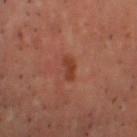Findings:
* notes: no biopsy performed (imaged during a skin exam)
* location: the head or neck
* acquisition: ~15 mm tile from a whole-body skin photo
* subject: male, about 55 years old